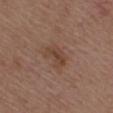| key | value |
|---|---|
| follow-up | imaged on a skin check; not biopsied |
| image source | total-body-photography crop, ~15 mm field of view |
| illumination | white-light illumination |
| site | the upper back |
| size | about 3.5 mm |
| patient | male, aged 68 to 72 |